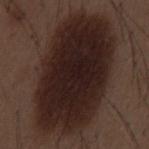The lesion was photographed on a routine skin check and not biopsied; there is no pathology result. Located on the mid back. About 13 mm across. Captured under white-light illumination. A male subject aged 48–52. A region of skin cropped from a whole-body photographic capture, roughly 15 mm wide. Automated image analysis of the tile measured a border-irregularity index near 1.5/10 and peripheral color asymmetry of about 1. And it measured a lesion-detection confidence of about 100/100.